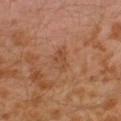The lesion was photographed on a routine skin check and not biopsied; there is no pathology result.
The recorded lesion diameter is about 3 mm.
Captured under cross-polarized illumination.
The lesion is located on the left leg.
A 15 mm close-up tile from a total-body photography series done for melanoma screening.
An algorithmic analysis of the crop reported a border-irregularity index near 3/10, a color-variation rating of about 3.5/10, and a peripheral color-asymmetry measure near 1.5. And it measured an automated nevus-likeness rating near 0 out of 100 and a lesion-detection confidence of about 100/100.
A male patient, aged 28 to 32.